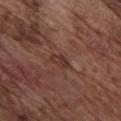This lesion was catalogued during total-body skin photography and was not selected for biopsy. The lesion is on the chest. Approximately 2.5 mm at its widest. Cropped from a total-body skin-imaging series; the visible field is about 15 mm. A male patient, about 75 years old. Imaged with white-light lighting.